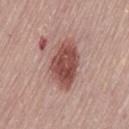– follow-up: imaged on a skin check; not biopsied
– location: the lower back
– lesion diameter: ~6 mm (longest diameter)
– acquisition: ~15 mm tile from a whole-body skin photo
– subject: female, aged 63–67